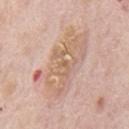| key | value |
|---|---|
| lighting | white-light |
| acquisition | total-body-photography crop, ~15 mm field of view |
| subject | male, roughly 75 years of age |
| size | ≈8.5 mm |
| location | the mid back |